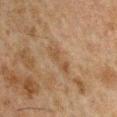Measured at roughly 4 mm in maximum diameter.
A 15 mm close-up extracted from a 3D total-body photography capture.
The patient is a male aged 48 to 52.
The tile uses cross-polarized illumination.
From the arm.